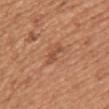biopsy status: catalogued during a skin exam; not biopsied
anatomic site: the upper back
subject: female, about 45 years old
image source: 15 mm crop, total-body photography
lighting: white-light illumination